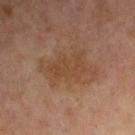The lesion was tiled from a total-body skin photograph and was not biopsied.
Located on the right upper arm.
A roughly 15 mm field-of-view crop from a total-body skin photograph.
A female patient, in their 60s.
The lesion's longest dimension is about 6.5 mm.
Captured under cross-polarized illumination.